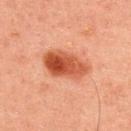The lesion was photographed on a routine skin check and not biopsied; there is no pathology result. The lesion's longest dimension is about 6 mm. A 15 mm crop from a total-body photograph taken for skin-cancer surveillance. The patient is a male in their mid-40s. Located on the upper back. The lesion-visualizer software estimated a nevus-likeness score of about 100/100. Imaged with cross-polarized lighting.